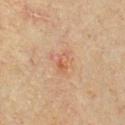Part of a total-body skin-imaging series; this lesion was reviewed on a skin check and was not flagged for biopsy. Measured at roughly 2.5 mm in maximum diameter. Captured under cross-polarized illumination. A region of skin cropped from a whole-body photographic capture, roughly 15 mm wide. Located on the chest. The patient is a male aged around 40. Automated image analysis of the tile measured a footprint of about 3 mm², an outline eccentricity of about 0.85 (0 = round, 1 = elongated), and a symmetry-axis asymmetry near 0.4. The software also gave a lesion color around L≈52 a*≈22 b*≈32 in CIELAB, about 7 CIELAB-L* units darker than the surrounding skin, and a lesion-to-skin contrast of about 5.5 (normalized; higher = more distinct).